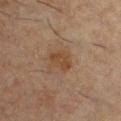Context:
The patient is a male in their 50s. An algorithmic analysis of the crop reported radial color variation of about 0.5. The software also gave an automated nevus-likeness rating near 10 out of 100. A 15 mm close-up tile from a total-body photography series done for melanoma screening. Captured under cross-polarized illumination. The recorded lesion diameter is about 3 mm. The lesion is located on the chest.